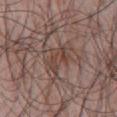The lesion was photographed on a routine skin check and not biopsied; there is no pathology result.
A 15 mm close-up extracted from a 3D total-body photography capture.
On the front of the torso.
A male patient aged 63 to 67.
Approximately 4 mm at its widest.
This is a white-light tile.
Automated image analysis of the tile measured two-axis asymmetry of about 0.4. The analysis additionally found a lesion color around L≈43 a*≈17 b*≈23 in CIELAB, a lesion–skin lightness drop of about 7, and a normalized border contrast of about 6. The analysis additionally found a border-irregularity index near 4.5/10 and internal color variation of about 4 on a 0–10 scale. The software also gave an automated nevus-likeness rating near 0 out of 100 and a detector confidence of about 85 out of 100 that the crop contains a lesion.